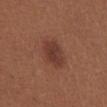This lesion was catalogued during total-body skin photography and was not selected for biopsy. Longest diameter approximately 4 mm. A roughly 15 mm field-of-view crop from a total-body skin photograph. A female subject, aged around 25. The tile uses white-light illumination. Located on the abdomen.